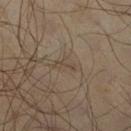This lesion was catalogued during total-body skin photography and was not selected for biopsy. Located on the right lower leg. Captured under cross-polarized illumination. The recorded lesion diameter is about 2.5 mm. A 15 mm close-up extracted from a 3D total-body photography capture. The subject is a male aged around 65.